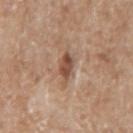Recorded during total-body skin imaging; not selected for excision or biopsy. A female patient in their 70s. From the left forearm. Cropped from a whole-body photographic skin survey; the tile spans about 15 mm.